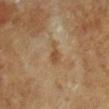Imaged during a routine full-body skin examination; the lesion was not biopsied and no histopathology is available. A male patient aged approximately 65. Captured under cross-polarized illumination. The lesion is located on the left lower leg. A roughly 15 mm field-of-view crop from a total-body skin photograph.